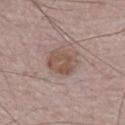The lesion was photographed on a routine skin check and not biopsied; there is no pathology result.
Approximately 4 mm at its widest.
The subject is a male in their mid-70s.
The lesion is located on the right lower leg.
A roughly 15 mm field-of-view crop from a total-body skin photograph.